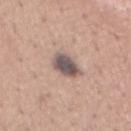biopsy_status: not biopsied; imaged during a skin examination
site: abdomen
lesion_size:
  long_diameter_mm_approx: 3.5
image:
  source: total-body photography crop
  field_of_view_mm: 15
patient:
  sex: male
  age_approx: 45
automated_metrics:
  area_mm2_approx: 6.5
  eccentricity: 0.8
  shape_asymmetry: 0.2
  border_irregularity_0_10: 2.0
  peripheral_color_asymmetry: 1.0